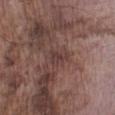Recorded during total-body skin imaging; not selected for excision or biopsy.
A 15 mm crop from a total-body photograph taken for skin-cancer surveillance.
The subject is a male in their mid- to late 70s.
Captured under white-light illumination.
Approximately 3 mm at its widest.
The lesion is on the left lower leg.